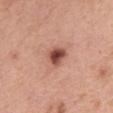Q: How was this image acquired?
A: ~15 mm tile from a whole-body skin photo
Q: How large is the lesion?
A: ~2.5 mm (longest diameter)
Q: Where on the body is the lesion?
A: the chest
Q: What lighting was used for the tile?
A: white-light
Q: Who is the patient?
A: female, roughly 45 years of age